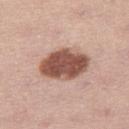follow-up: imaged on a skin check; not biopsied
body site: the right thigh
image source: total-body-photography crop, ~15 mm field of view
subject: female, aged 28 to 32